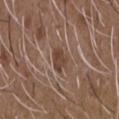From the chest. Cropped from a whole-body photographic skin survey; the tile spans about 15 mm. The patient is a male aged 48 to 52. The total-body-photography lesion software estimated a footprint of about 4.5 mm², a shape eccentricity near 0.65, and a shape-asymmetry score of about 0.2 (0 = symmetric). About 3 mm across.